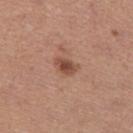Background: A 15 mm crop from a total-body photograph taken for skin-cancer surveillance. Approximately 3 mm at its widest. A female patient, aged approximately 35. The total-body-photography lesion software estimated an area of roughly 5 mm², a shape eccentricity near 0.75, and two-axis asymmetry of about 0.25. And it measured a classifier nevus-likeness of about 85/100 and a lesion-detection confidence of about 100/100. The lesion is located on the left thigh.